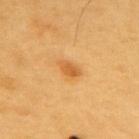This lesion was catalogued during total-body skin photography and was not selected for biopsy. This is a cross-polarized tile. Measured at roughly 3 mm in maximum diameter. An algorithmic analysis of the crop reported a lesion area of about 4 mm², a shape eccentricity near 0.8, and a symmetry-axis asymmetry near 0.25. It also reported a border-irregularity index near 2.5/10 and internal color variation of about 2.5 on a 0–10 scale. It also reported a nevus-likeness score of about 90/100 and lesion-presence confidence of about 100/100. A lesion tile, about 15 mm wide, cut from a 3D total-body photograph. The lesion is located on the upper back. A male patient approximately 60 years of age.